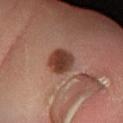| key | value |
|---|---|
| follow-up | catalogued during a skin exam; not biopsied |
| acquisition | ~15 mm tile from a whole-body skin photo |
| patient | female, aged 33 to 37 |
| image-analysis metrics | a lesion area of about 8.5 mm² and an outline eccentricity of about 0.4 (0 = round, 1 = elongated); a lesion color around L≈39 a*≈22 b*≈27 in CIELAB |
| tile lighting | cross-polarized |
| anatomic site | the left lower leg |